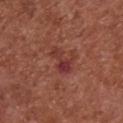{
  "biopsy_status": "not biopsied; imaged during a skin examination",
  "site": "chest",
  "lesion_size": {
    "long_diameter_mm_approx": 3.5
  },
  "lighting": "white-light",
  "image": {
    "source": "total-body photography crop",
    "field_of_view_mm": 15
  },
  "patient": {
    "sex": "female",
    "age_approx": 75
  }
}